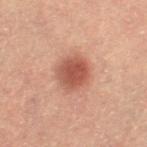Clinical impression: Recorded during total-body skin imaging; not selected for excision or biopsy. Acquisition and patient details: Imaged with cross-polarized lighting. From the right thigh. The subject is a female aged 58 to 62. Measured at roughly 4 mm in maximum diameter. A 15 mm crop from a total-body photograph taken for skin-cancer surveillance.